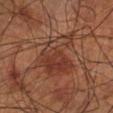biopsy status = catalogued during a skin exam; not biopsied
acquisition = ~15 mm crop, total-body skin-cancer survey
diameter = ≈6 mm
location = the left lower leg
patient = male, aged around 70
automated lesion analysis = an area of roughly 19 mm², a shape eccentricity near 0.55, and a symmetry-axis asymmetry near 0.45; a border-irregularity index near 6/10 and radial color variation of about 2; an automated nevus-likeness rating near 40 out of 100 and a lesion-detection confidence of about 100/100